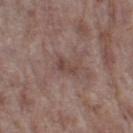The lesion was photographed on a routine skin check and not biopsied; there is no pathology result.